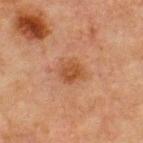Q: What are the patient's age and sex?
A: male, about 65 years old
Q: How was this image acquired?
A: total-body-photography crop, ~15 mm field of view
Q: What is the anatomic site?
A: the front of the torso
Q: Lesion size?
A: ≈3 mm